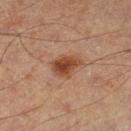<lesion>
  <image>
    <source>total-body photography crop</source>
    <field_of_view_mm>15</field_of_view_mm>
  </image>
  <site>left lower leg</site>
  <patient>
    <sex>male</sex>
    <age_approx>65</age_approx>
  </patient>
</lesion>